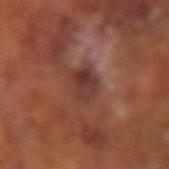Assessment: Captured during whole-body skin photography for melanoma surveillance; the lesion was not biopsied. Image and clinical context: Cropped from a whole-body photographic skin survey; the tile spans about 15 mm. Located on the left arm. The subject is a male aged 63–67. The lesion's longest dimension is about 3.5 mm. An algorithmic analysis of the crop reported an area of roughly 6.5 mm² and an outline eccentricity of about 0.7 (0 = round, 1 = elongated). And it measured an average lesion color of about L≈34 a*≈21 b*≈23 (CIELAB), roughly 8 lightness units darker than nearby skin, and a normalized lesion–skin contrast near 8. The software also gave a lesion-detection confidence of about 95/100.